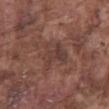Part of a total-body skin-imaging series; this lesion was reviewed on a skin check and was not flagged for biopsy. On the abdomen. The lesion-visualizer software estimated an area of roughly 10 mm² and a shape-asymmetry score of about 0.4 (0 = symmetric). The analysis additionally found a mean CIELAB color near L≈39 a*≈19 b*≈22, about 6 CIELAB-L* units darker than the surrounding skin, and a normalized lesion–skin contrast near 5.5. And it measured border irregularity of about 4.5 on a 0–10 scale, internal color variation of about 4.5 on a 0–10 scale, and a peripheral color-asymmetry measure near 1.5. The analysis additionally found a classifier nevus-likeness of about 0/100 and a detector confidence of about 80 out of 100 that the crop contains a lesion. Measured at roughly 4 mm in maximum diameter. A lesion tile, about 15 mm wide, cut from a 3D total-body photograph. The tile uses white-light illumination. The patient is a male aged 73–77.